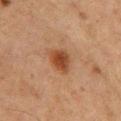Case summary:
* biopsy status: no biopsy performed (imaged during a skin exam)
* automated lesion analysis: an area of roughly 6.5 mm² and two-axis asymmetry of about 0.25
* patient: male, aged around 75
* anatomic site: the chest
* illumination: cross-polarized illumination
* lesion size: ~3.5 mm (longest diameter)
* acquisition: ~15 mm tile from a whole-body skin photo